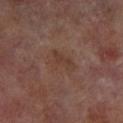Findings:
* follow-up: imaged on a skin check; not biopsied
* tile lighting: cross-polarized
* location: the right lower leg
* subject: male, aged 68–72
* size: about 3.5 mm
* image source: ~15 mm crop, total-body skin-cancer survey